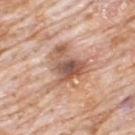Notes:
• workup — imaged on a skin check; not biopsied
• lesion size — about 5.5 mm
• acquisition — ~15 mm crop, total-body skin-cancer survey
• tile lighting — white-light illumination
• automated metrics — a footprint of about 17 mm², a shape eccentricity near 0.35, and a shape-asymmetry score of about 0.45 (0 = symmetric); an automated nevus-likeness rating near 5 out of 100
• subject — male, aged 78–82
• location — the upper back